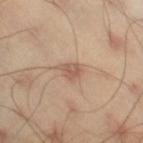Q: Is there a histopathology result?
A: total-body-photography surveillance lesion; no biopsy
Q: Patient demographics?
A: male, aged around 45
Q: Illumination type?
A: cross-polarized illumination
Q: Lesion location?
A: the right thigh
Q: How large is the lesion?
A: ~2.5 mm (longest diameter)
Q: How was this image acquired?
A: 15 mm crop, total-body photography